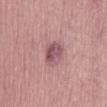Findings:
* notes — no biopsy performed (imaged during a skin exam)
* site — the front of the torso
* lighting — white-light illumination
* patient — male, aged approximately 40
* image — 15 mm crop, total-body photography
* automated metrics — a lesion area of about 7 mm²; a lesion color around L≈53 a*≈24 b*≈17 in CIELAB and a lesion-to-skin contrast of about 7.5 (normalized; higher = more distinct)